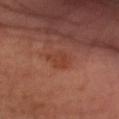Part of a total-body skin-imaging series; this lesion was reviewed on a skin check and was not flagged for biopsy.
The lesion-visualizer software estimated roughly 5 lightness units darker than nearby skin and a normalized border contrast of about 5. And it measured a border-irregularity index near 2/10, a within-lesion color-variation index near 2/10, and peripheral color asymmetry of about 0.5. It also reported a nevus-likeness score of about 0/100 and lesion-presence confidence of about 100/100.
A region of skin cropped from a whole-body photographic capture, roughly 15 mm wide.
Located on the right forearm.
A male subject aged approximately 50.
The lesion's longest dimension is about 3.5 mm.
Captured under cross-polarized illumination.